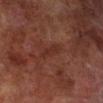anatomic site: the right lower leg
illumination: cross-polarized
image: total-body-photography crop, ~15 mm field of view
TBP lesion metrics: a mean CIELAB color near L≈24 a*≈19 b*≈21, roughly 4 lightness units darker than nearby skin, and a lesion-to-skin contrast of about 5 (normalized; higher = more distinct); internal color variation of about 0 on a 0–10 scale and a peripheral color-asymmetry measure near 0; an automated nevus-likeness rating near 0 out of 100 and a lesion-detection confidence of about 100/100
patient: male, aged 68 to 72
lesion diameter: ≈3.5 mm